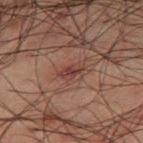This lesion was catalogued during total-body skin photography and was not selected for biopsy. The patient is a male in their 50s. A 15 mm close-up tile from a total-body photography series done for melanoma screening. From the right thigh.